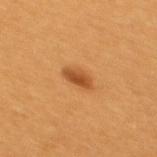| feature | finding |
|---|---|
| biopsy status | catalogued during a skin exam; not biopsied |
| illumination | cross-polarized illumination |
| automated lesion analysis | a lesion color around L≈47 a*≈25 b*≈40 in CIELAB, roughly 11 lightness units darker than nearby skin, and a lesion-to-skin contrast of about 8 (normalized; higher = more distinct) |
| body site | the upper back |
| diameter | ~3 mm (longest diameter) |
| acquisition | ~15 mm tile from a whole-body skin photo |
| subject | female, in their 30s |